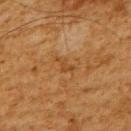Impression:
Recorded during total-body skin imaging; not selected for excision or biopsy.